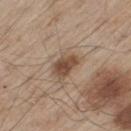Notes:
– workup · total-body-photography surveillance lesion; no biopsy
– anatomic site · the left thigh
– patient · male, about 60 years old
– imaging modality · ~15 mm tile from a whole-body skin photo